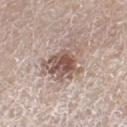  biopsy_status: not biopsied; imaged during a skin examination
  lighting: white-light
  image:
    source: total-body photography crop
    field_of_view_mm: 15
  site: left thigh
  patient:
    sex: male
    age_approx: 80